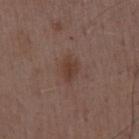Case summary:
– follow-up — total-body-photography surveillance lesion; no biopsy